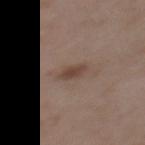No biopsy was performed on this lesion — it was imaged during a full skin examination and was not determined to be concerning. Longest diameter approximately 2.5 mm. A 15 mm crop from a total-body photograph taken for skin-cancer surveillance. The patient is a male in their 50s. Located on the upper back. The tile uses white-light illumination. The lesion-visualizer software estimated a lesion area of about 4 mm², a shape eccentricity near 0.75, and two-axis asymmetry of about 0.3. The software also gave a mean CIELAB color near L≈43 a*≈16 b*≈24 and about 8 CIELAB-L* units darker than the surrounding skin. It also reported a border-irregularity index near 2.5/10, a within-lesion color-variation index near 1.5/10, and radial color variation of about 0.5. The analysis additionally found an automated nevus-likeness rating near 35 out of 100 and a lesion-detection confidence of about 100/100.